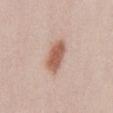This lesion was catalogued during total-body skin photography and was not selected for biopsy. A female subject, roughly 40 years of age. This image is a 15 mm lesion crop taken from a total-body photograph. Measured at roughly 4.5 mm in maximum diameter. Located on the front of the torso.